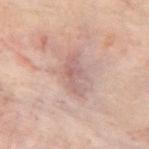<lesion>
  <lighting>cross-polarized</lighting>
  <image>
    <source>total-body photography crop</source>
    <field_of_view_mm>15</field_of_view_mm>
  </image>
  <automated_metrics>
    <cielab_L>49</cielab_L>
    <cielab_a>16</cielab_a>
    <cielab_b>19</cielab_b>
    <vs_skin_contrast_norm>5.5</vs_skin_contrast_norm>
  </automated_metrics>
  <site>back</site>
  <lesion_size>
    <long_diameter_mm_approx>4.5</long_diameter_mm_approx>
  </lesion_size>
  <patient>
    <sex>male</sex>
    <age_approx>50</age_approx>
  </patient>
</lesion>